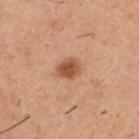Captured during whole-body skin photography for melanoma surveillance; the lesion was not biopsied. The lesion is located on the back. A 15 mm crop from a total-body photograph taken for skin-cancer surveillance. The lesion's longest dimension is about 3 mm. A male patient, in their 40s.